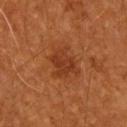- follow-up · imaged on a skin check; not biopsied
- subject · male, approximately 60 years of age
- imaging modality · 15 mm crop, total-body photography
- body site · the upper back
- diameter · ≈4 mm
- lighting · cross-polarized illumination